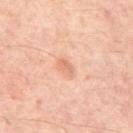  biopsy_status: not biopsied; imaged during a skin examination
  automated_metrics:
    cielab_L: 64
    cielab_a: 24
    cielab_b: 31
    vs_skin_darker_L: 9.0
    vs_skin_contrast_norm: 5.5
    color_variation_0_10: 0.0
    peripheral_color_asymmetry: 0.0
    nevus_likeness_0_100: 80
    lesion_detection_confidence_0_100: 100
  lesion_size:
    long_diameter_mm_approx: 2.0
  site: mid back
  image:
    source: total-body photography crop
    field_of_view_mm: 15
  patient:
    sex: male
    age_approx: 65